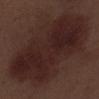Notes:
– notes · catalogued during a skin exam; not biopsied
– site · the left lower leg
– size · ~13.5 mm (longest diameter)
– imaging modality · ~15 mm tile from a whole-body skin photo
– patient · male, aged 68–72